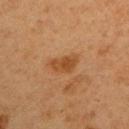{"biopsy_status": "not biopsied; imaged during a skin examination", "lesion_size": {"long_diameter_mm_approx": 4.0}, "automated_metrics": {"area_mm2_approx": 6.5, "eccentricity": 0.8, "shape_asymmetry": 0.35, "border_irregularity_0_10": 3.5, "peripheral_color_asymmetry": 1.0, "nevus_likeness_0_100": 75, "lesion_detection_confidence_0_100": 100}, "site": "left upper arm", "image": {"source": "total-body photography crop", "field_of_view_mm": 15}, "patient": {"sex": "female", "age_approx": 40}, "lighting": "cross-polarized"}